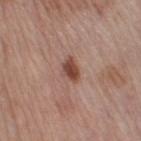<case>
<biopsy_status>not biopsied; imaged during a skin examination</biopsy_status>
<image>
  <source>total-body photography crop</source>
  <field_of_view_mm>15</field_of_view_mm>
</image>
<patient>
  <sex>female</sex>
  <age_approx>50</age_approx>
</patient>
<automated_metrics>
  <area_mm2_approx>4.5</area_mm2_approx>
  <eccentricity>0.8</eccentricity>
  <shape_asymmetry>0.25</shape_asymmetry>
  <border_irregularity_0_10>2.5</border_irregularity_0_10>
  <color_variation_0_10>2.5</color_variation_0_10>
  <peripheral_color_asymmetry>0.5</peripheral_color_asymmetry>
  <nevus_likeness_0_100>95</nevus_likeness_0_100>
  <lesion_detection_confidence_0_100>100</lesion_detection_confidence_0_100>
</automated_metrics>
<lighting>white-light</lighting>
<lesion_size>
  <long_diameter_mm_approx>3.0</long_diameter_mm_approx>
</lesion_size>
<site>leg</site>
</case>